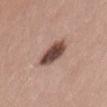{"biopsy_status": "not biopsied; imaged during a skin examination", "site": "lower back", "patient": {"sex": "female", "age_approx": 35}, "image": {"source": "total-body photography crop", "field_of_view_mm": 15}}